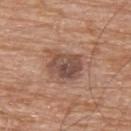biopsy status: total-body-photography surveillance lesion; no biopsy
acquisition: ~15 mm crop, total-body skin-cancer survey
anatomic site: the upper back
lighting: white-light illumination
subject: male, aged 78–82